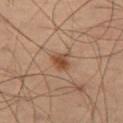This lesion was catalogued during total-body skin photography and was not selected for biopsy. A roughly 15 mm field-of-view crop from a total-body skin photograph. A male subject, aged approximately 55. On the left thigh. An algorithmic analysis of the crop reported a mean CIELAB color near L≈38 a*≈16 b*≈26, about 8 CIELAB-L* units darker than the surrounding skin, and a normalized lesion–skin contrast near 7.5. The analysis additionally found a border-irregularity index near 2.5/10, internal color variation of about 3 on a 0–10 scale, and a peripheral color-asymmetry measure near 1. About 2.5 mm across. Imaged with cross-polarized lighting.